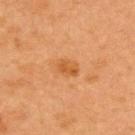workup = no biopsy performed (imaged during a skin exam); imaging modality = ~15 mm crop, total-body skin-cancer survey; patient = female, aged around 40; site = the upper back.